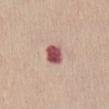The lesion was tiled from a total-body skin photograph and was not biopsied.
A female subject aged around 40.
The lesion is located on the abdomen.
A 15 mm crop from a total-body photograph taken for skin-cancer surveillance.
The tile uses white-light illumination.
The recorded lesion diameter is about 3 mm.